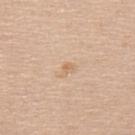Q: Is there a histopathology result?
A: no biopsy performed (imaged during a skin exam)
Q: Who is the patient?
A: female, in their mid-50s
Q: Lesion location?
A: the upper back
Q: How was this image acquired?
A: total-body-photography crop, ~15 mm field of view
Q: How large is the lesion?
A: ≈1 mm
Q: Automated lesion metrics?
A: border irregularity of about 4.5 on a 0–10 scale, a color-variation rating of about 0/10, and radial color variation of about 0; lesion-presence confidence of about 100/100